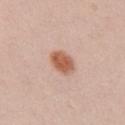This lesion was catalogued during total-body skin photography and was not selected for biopsy.
A 15 mm close-up tile from a total-body photography series done for melanoma screening.
The lesion is on the right upper arm.
The subject is a female aged 23–27.